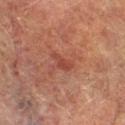Findings:
- notes — total-body-photography surveillance lesion; no biopsy
- imaging modality — ~15 mm tile from a whole-body skin photo
- location — the left lower leg
- subject — male, aged around 75